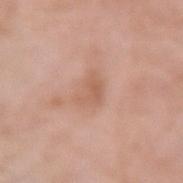Clinical summary:
The recorded lesion diameter is about 3 mm. From the arm. A 15 mm close-up tile from a total-body photography series done for melanoma screening. Captured under white-light illumination. A female subject, about 75 years old. Automated tile analysis of the lesion measured an eccentricity of roughly 0.7 and two-axis asymmetry of about 0.4. The analysis additionally found a mean CIELAB color near L≈60 a*≈21 b*≈30 and a normalized border contrast of about 5.5. It also reported a border-irregularity rating of about 4/10, a within-lesion color-variation index near 2.5/10, and a peripheral color-asymmetry measure near 1.